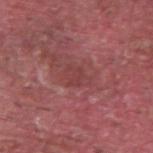* workup · imaged on a skin check; not biopsied
* lesion size · ≈2.5 mm
* site · the right upper arm
* subject · male, in their 40s
* acquisition · total-body-photography crop, ~15 mm field of view
* tile lighting · white-light